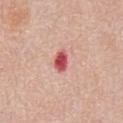Impression: No biopsy was performed on this lesion — it was imaged during a full skin examination and was not determined to be concerning. Image and clinical context: This is a white-light tile. This image is a 15 mm lesion crop taken from a total-body photograph. A male patient, approximately 80 years of age. Located on the abdomen. Approximately 2.5 mm at its widest. The lesion-visualizer software estimated an area of roughly 4 mm² and a shape-asymmetry score of about 0.25 (0 = symmetric). The analysis additionally found a mean CIELAB color near L≈54 a*≈38 b*≈25, roughly 17 lightness units darker than nearby skin, and a lesion-to-skin contrast of about 11 (normalized; higher = more distinct).